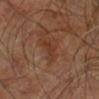| key | value |
|---|---|
| acquisition | 15 mm crop, total-body photography |
| image-analysis metrics | a color-variation rating of about 1/10 and a peripheral color-asymmetry measure near 0; a classifier nevus-likeness of about 0/100 |
| site | the right leg |
| subject | male, in their 60s |
| tile lighting | cross-polarized |
| size | ≈4 mm |